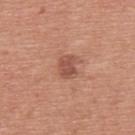The lesion was photographed on a routine skin check and not biopsied; there is no pathology result.
From the upper back.
A male subject, aged approximately 55.
A region of skin cropped from a whole-body photographic capture, roughly 15 mm wide.